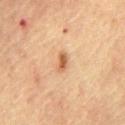Captured during whole-body skin photography for melanoma surveillance; the lesion was not biopsied.
A region of skin cropped from a whole-body photographic capture, roughly 15 mm wide.
The patient is a male roughly 65 years of age.
The lesion's longest dimension is about 2.5 mm.
Located on the chest.
Captured under cross-polarized illumination.